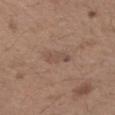The lesion is on the left forearm. About 3 mm across. A male patient aged approximately 70. The tile uses white-light illumination. A roughly 15 mm field-of-view crop from a total-body skin photograph. Automated tile analysis of the lesion measured a classifier nevus-likeness of about 0/100 and a detector confidence of about 100 out of 100 that the crop contains a lesion.